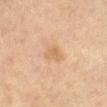This lesion was catalogued during total-body skin photography and was not selected for biopsy. Cropped from a total-body skin-imaging series; the visible field is about 15 mm. On the arm. Imaged with cross-polarized lighting. A female patient approximately 60 years of age.